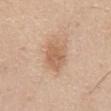biopsy_status: not biopsied; imaged during a skin examination
image:
  source: total-body photography crop
  field_of_view_mm: 15
patient:
  sex: male
  age_approx: 40
lighting: white-light
site: front of the torso
lesion_size:
  long_diameter_mm_approx: 4.0